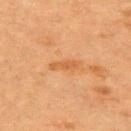<record>
<site>upper back</site>
<automated_metrics>
  <lesion_detection_confidence_0_100>100</lesion_detection_confidence_0_100>
</automated_metrics>
<image>
  <source>total-body photography crop</source>
  <field_of_view_mm>15</field_of_view_mm>
</image>
<patient>
  <sex>female</sex>
  <age_approx>55</age_approx>
</patient>
<lesion_size>
  <long_diameter_mm_approx>3.5</long_diameter_mm_approx>
</lesion_size>
<lighting>cross-polarized</lighting>
</record>